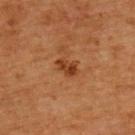Q: Is there a histopathology result?
A: total-body-photography surveillance lesion; no biopsy
Q: How large is the lesion?
A: ~2.5 mm (longest diameter)
Q: Patient demographics?
A: male, roughly 65 years of age
Q: How was this image acquired?
A: ~15 mm tile from a whole-body skin photo
Q: Where on the body is the lesion?
A: the upper back
Q: Illumination type?
A: cross-polarized illumination